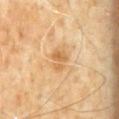Recorded during total-body skin imaging; not selected for excision or biopsy.
A close-up tile cropped from a whole-body skin photograph, about 15 mm across.
Imaged with cross-polarized lighting.
Located on the abdomen.
A male subject, aged 58–62.